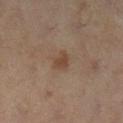Clinical impression:
Captured during whole-body skin photography for melanoma surveillance; the lesion was not biopsied.
Context:
The patient is a female in their 50s. This is a cross-polarized tile. Located on the left lower leg. A region of skin cropped from a whole-body photographic capture, roughly 15 mm wide.